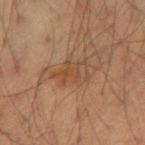Part of a total-body skin-imaging series; this lesion was reviewed on a skin check and was not flagged for biopsy. A male patient, in their mid-30s. Located on the right upper arm. About 4 mm across. An algorithmic analysis of the crop reported a lesion area of about 7.5 mm², a shape eccentricity near 0.75, and a shape-asymmetry score of about 0.4 (0 = symmetric). A 15 mm crop from a total-body photograph taken for skin-cancer surveillance. This is a cross-polarized tile.